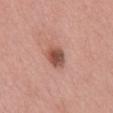Assessment: Recorded during total-body skin imaging; not selected for excision or biopsy. Clinical summary: Captured under white-light illumination. The total-body-photography lesion software estimated a lesion color around L≈51 a*≈24 b*≈27 in CIELAB, a lesion–skin lightness drop of about 14, and a normalized lesion–skin contrast near 9. It also reported a border-irregularity index near 1.5/10, internal color variation of about 5.5 on a 0–10 scale, and peripheral color asymmetry of about 2. It also reported a classifier nevus-likeness of about 65/100 and a detector confidence of about 100 out of 100 that the crop contains a lesion. A 15 mm crop from a total-body photograph taken for skin-cancer surveillance. A female patient, about 70 years old. About 3 mm across. The lesion is on the mid back.